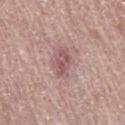The lesion was photographed on a routine skin check and not biopsied; there is no pathology result. The recorded lesion diameter is about 3.5 mm. A lesion tile, about 15 mm wide, cut from a 3D total-body photograph. A male subject about 70 years old. The lesion is on the leg.